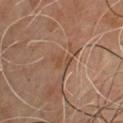<lesion>
<biopsy_status>not biopsied; imaged during a skin examination</biopsy_status>
<patient>
  <sex>male</sex>
  <age_approx>65</age_approx>
</patient>
<lesion_size>
  <long_diameter_mm_approx>2.5</long_diameter_mm_approx>
</lesion_size>
<lighting>cross-polarized</lighting>
<site>chest</site>
<image>
  <source>total-body photography crop</source>
  <field_of_view_mm>15</field_of_view_mm>
</image>
</lesion>